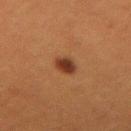workup: catalogued during a skin exam; not biopsied | subject: male, aged 38–42 | anatomic site: the arm | image: ~15 mm tile from a whole-body skin photo.